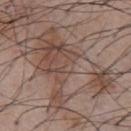Part of a total-body skin-imaging series; this lesion was reviewed on a skin check and was not flagged for biopsy.
On the front of the torso.
The lesion-visualizer software estimated a lesion area of about 45 mm² and a shape-asymmetry score of about 0.7 (0 = symmetric).
Longest diameter approximately 12 mm.
A 15 mm crop from a total-body photograph taken for skin-cancer surveillance.
The patient is a male about 55 years old.
The tile uses white-light illumination.